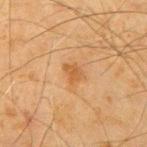Assessment: Imaged during a routine full-body skin examination; the lesion was not biopsied and no histopathology is available. Clinical summary: The tile uses cross-polarized illumination. On the left upper arm. Longest diameter approximately 3 mm. Cropped from a whole-body photographic skin survey; the tile spans about 15 mm. The subject is a male aged around 70.